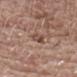Assessment: This lesion was catalogued during total-body skin photography and was not selected for biopsy. Image and clinical context: A 15 mm close-up tile from a total-body photography series done for melanoma screening. A male patient roughly 80 years of age. The lesion is located on the chest. About 2.5 mm across. The tile uses white-light illumination. The lesion-visualizer software estimated a lesion area of about 3.5 mm² and an eccentricity of roughly 0.8. It also reported a normalized lesion–skin contrast near 7.5. And it measured a border-irregularity rating of about 3/10 and a within-lesion color-variation index near 5/10. The software also gave a classifier nevus-likeness of about 0/100 and a detector confidence of about 100 out of 100 that the crop contains a lesion.